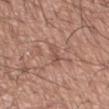Q: Was this lesion biopsied?
A: no biopsy performed (imaged during a skin exam)
Q: How was this image acquired?
A: total-body-photography crop, ~15 mm field of view
Q: Patient demographics?
A: male, in their mid-70s
Q: Where on the body is the lesion?
A: the right forearm